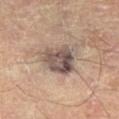{"lighting": "cross-polarized", "automated_metrics": {"cielab_L": 49, "cielab_a": 14, "cielab_b": 20, "vs_skin_darker_L": 14.0, "vs_skin_contrast_norm": 11.0, "border_irregularity_0_10": 2.5, "color_variation_0_10": 8.5, "peripheral_color_asymmetry": 3.0}, "patient": {"sex": "male", "age_approx": 70}, "image": {"source": "total-body photography crop", "field_of_view_mm": 15}, "site": "right thigh", "lesion_size": {"long_diameter_mm_approx": 4.0}}